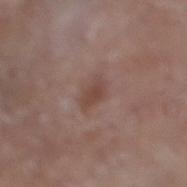Clinical impression: This lesion was catalogued during total-body skin photography and was not selected for biopsy. Acquisition and patient details: Cropped from a whole-body photographic skin survey; the tile spans about 15 mm. An algorithmic analysis of the crop reported border irregularity of about 3 on a 0–10 scale and a color-variation rating of about 1.5/10. The software also gave a nevus-likeness score of about 5/100. About 3 mm across. The tile uses white-light illumination. A male patient, aged approximately 80. On the right lower leg.